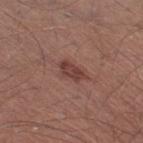A male subject, aged around 55. The lesion is on the right thigh. The lesion's longest dimension is about 3 mm. A 15 mm close-up tile from a total-body photography series done for melanoma screening. This is a white-light tile.